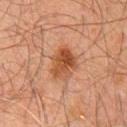workup = imaged on a skin check; not biopsied
patient = male, aged approximately 60
illumination = cross-polarized
image = 15 mm crop, total-body photography
location = the chest
diameter = about 4 mm